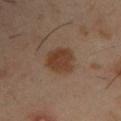Part of a total-body skin-imaging series; this lesion was reviewed on a skin check and was not flagged for biopsy.
A male subject, about 40 years old.
From the right upper arm.
A 15 mm close-up tile from a total-body photography series done for melanoma screening.